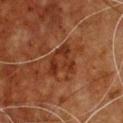site: the chest | TBP lesion metrics: a lesion area of about 9 mm², an outline eccentricity of about 0.7 (0 = round, 1 = elongated), and two-axis asymmetry of about 0.45; a border-irregularity index near 5/10, a within-lesion color-variation index near 3.5/10, and radial color variation of about 1; a classifier nevus-likeness of about 0/100 and a lesion-detection confidence of about 100/100 | imaging modality: ~15 mm crop, total-body skin-cancer survey | patient: male, aged approximately 60 | tile lighting: cross-polarized illumination.